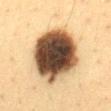{"biopsy_status": "not biopsied; imaged during a skin examination", "automated_metrics": {"area_mm2_approx": 37.0, "eccentricity": 0.35, "shape_asymmetry": 0.1, "border_irregularity_0_10": 1.5, "nevus_likeness_0_100": 5, "lesion_detection_confidence_0_100": 100}, "lighting": "cross-polarized", "image": {"source": "total-body photography crop", "field_of_view_mm": 15}, "patient": {"sex": "female", "age_approx": 40}, "site": "mid back"}